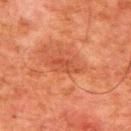lighting: cross-polarized | imaging modality: 15 mm crop, total-body photography | image-analysis metrics: a lesion color around L≈40 a*≈28 b*≈32 in CIELAB, roughly 6 lightness units darker than nearby skin, and a normalized border contrast of about 5.5; border irregularity of about 4.5 on a 0–10 scale, a color-variation rating of about 1.5/10, and a peripheral color-asymmetry measure near 0.5 | diameter: ~3.5 mm (longest diameter) | location: the upper back | subject: male, aged 78–82.